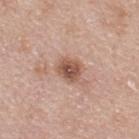{"biopsy_status": "not biopsied; imaged during a skin examination", "lesion_size": {"long_diameter_mm_approx": 3.0}, "site": "back", "image": {"source": "total-body photography crop", "field_of_view_mm": 15}, "patient": {"sex": "female", "age_approx": 55}, "lighting": "white-light", "automated_metrics": {"vs_skin_darker_L": 13.0, "vs_skin_contrast_norm": 9.0, "nevus_likeness_0_100": 80, "lesion_detection_confidence_0_100": 100}}